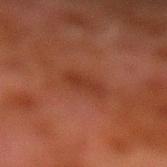Impression: Imaged during a routine full-body skin examination; the lesion was not biopsied and no histopathology is available. Image and clinical context: This is a cross-polarized tile. The recorded lesion diameter is about 3.5 mm. The patient is a male aged 78 to 82. The lesion is located on the left lower leg. A 15 mm close-up tile from a total-body photography series done for melanoma screening.